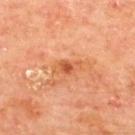Impression: No biopsy was performed on this lesion — it was imaged during a full skin examination and was not determined to be concerning. Image and clinical context: The lesion is on the upper back. This is a cross-polarized tile. A male subject, roughly 65 years of age. Approximately 3 mm at its widest. A 15 mm close-up extracted from a 3D total-body photography capture.